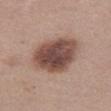Captured during whole-body skin photography for melanoma surveillance; the lesion was not biopsied. Cropped from a total-body skin-imaging series; the visible field is about 15 mm. The lesion is on the abdomen. A female patient aged 38–42.